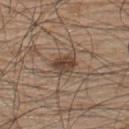- notes: total-body-photography surveillance lesion; no biopsy
- size: ≈3 mm
- subject: male, aged around 50
- site: the back
- illumination: white-light illumination
- image source: total-body-photography crop, ~15 mm field of view